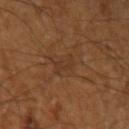Impression:
Part of a total-body skin-imaging series; this lesion was reviewed on a skin check and was not flagged for biopsy.
Context:
Captured under cross-polarized illumination. Measured at roughly 3 mm in maximum diameter. A 15 mm crop from a total-body photograph taken for skin-cancer surveillance. The lesion is located on the arm. The subject is a male in their 60s. Automated image analysis of the tile measured a footprint of about 3.5 mm², an eccentricity of roughly 0.9, and a symmetry-axis asymmetry near 0.35. And it measured an average lesion color of about L≈31 a*≈17 b*≈27 (CIELAB), roughly 5 lightness units darker than nearby skin, and a normalized lesion–skin contrast near 4.5. And it measured border irregularity of about 5 on a 0–10 scale, a color-variation rating of about 0/10, and peripheral color asymmetry of about 0. The analysis additionally found an automated nevus-likeness rating near 0 out of 100 and lesion-presence confidence of about 80/100.